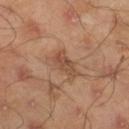Located on the right lower leg. Captured under cross-polarized illumination. The subject is a male about 45 years old. Automated image analysis of the tile measured an eccentricity of roughly 0.8. It also reported a mean CIELAB color near L≈48 a*≈20 b*≈30, roughly 9 lightness units darker than nearby skin, and a lesion-to-skin contrast of about 6.5 (normalized; higher = more distinct). The analysis additionally found a border-irregularity index near 3/10, a color-variation rating of about 4/10, and peripheral color asymmetry of about 1.5. It also reported a classifier nevus-likeness of about 5/100 and lesion-presence confidence of about 100/100. Measured at roughly 3.5 mm in maximum diameter. A lesion tile, about 15 mm wide, cut from a 3D total-body photograph.